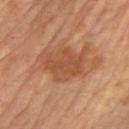Q: Is there a histopathology result?
A: no biopsy performed (imaged during a skin exam)
Q: How large is the lesion?
A: ≈5.5 mm
Q: What did automated image analysis measure?
A: a border-irregularity index near 3/10, a within-lesion color-variation index near 2.5/10, and peripheral color asymmetry of about 1; an automated nevus-likeness rating near 0 out of 100 and a lesion-detection confidence of about 100/100
Q: What is the imaging modality?
A: total-body-photography crop, ~15 mm field of view
Q: What is the anatomic site?
A: the left upper arm
Q: What lighting was used for the tile?
A: cross-polarized illumination
Q: Patient demographics?
A: female, in their mid- to late 60s